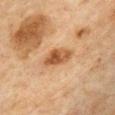biopsy_status: not biopsied; imaged during a skin examination
patient:
  sex: male
  age_approx: 65
site: front of the torso
lesion_size:
  long_diameter_mm_approx: 4.0
lighting: cross-polarized
image:
  source: total-body photography crop
  field_of_view_mm: 15